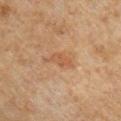Recorded during total-body skin imaging; not selected for excision or biopsy. The subject is a male aged 48–52. Located on the chest. The recorded lesion diameter is about 3.5 mm. A region of skin cropped from a whole-body photographic capture, roughly 15 mm wide.